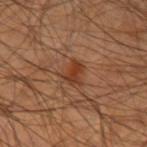Case summary:
- follow-up — total-body-photography surveillance lesion; no biopsy
- size — ≈3 mm
- lighting — cross-polarized illumination
- image source — ~15 mm crop, total-body skin-cancer survey
- body site — the left upper arm
- subject — male, roughly 45 years of age